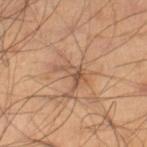{
  "patient": {
    "sex": "male",
    "age_approx": 40
  },
  "image": {
    "source": "total-body photography crop",
    "field_of_view_mm": 15
  },
  "site": "right lower leg",
  "lesion_size": {
    "long_diameter_mm_approx": 3.5
  },
  "lighting": "cross-polarized"
}